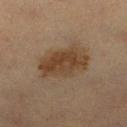follow-up: no biopsy performed (imaged during a skin exam) | acquisition: 15 mm crop, total-body photography | size: about 6 mm | automated lesion analysis: an area of roughly 17 mm², an outline eccentricity of about 0.75 (0 = round, 1 = elongated), and two-axis asymmetry of about 0.2; an automated nevus-likeness rating near 60 out of 100 and a lesion-detection confidence of about 100/100 | body site: the right lower leg | subject: female, roughly 40 years of age.